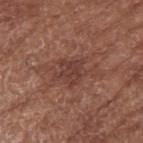Q: Was a biopsy performed?
A: imaged on a skin check; not biopsied
Q: How was this image acquired?
A: total-body-photography crop, ~15 mm field of view
Q: Patient demographics?
A: female, in their mid- to late 70s
Q: How was the tile lit?
A: white-light
Q: What is the anatomic site?
A: the right forearm